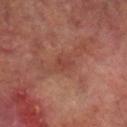{"biopsy_status": "not biopsied; imaged during a skin examination", "patient": {"sex": "male", "age_approx": 70}, "image": {"source": "total-body photography crop", "field_of_view_mm": 15}, "site": "right lower leg", "lesion_size": {"long_diameter_mm_approx": 2.5}}